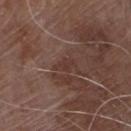Recorded during total-body skin imaging; not selected for excision or biopsy.
Imaged with white-light lighting.
Measured at roughly 2.5 mm in maximum diameter.
The lesion is located on the chest.
A male subject in their 80s.
Cropped from a total-body skin-imaging series; the visible field is about 15 mm.